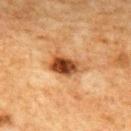Q: Was a biopsy performed?
A: no biopsy performed (imaged during a skin exam)
Q: Lesion size?
A: about 4 mm
Q: Patient demographics?
A: female, aged 58 to 62
Q: What kind of image is this?
A: ~15 mm crop, total-body skin-cancer survey
Q: Automated lesion metrics?
A: a footprint of about 8.5 mm² and two-axis asymmetry of about 0.3; an average lesion color of about L≈40 a*≈22 b*≈34 (CIELAB), a lesion–skin lightness drop of about 15, and a normalized border contrast of about 11.5; a border-irregularity index near 3/10, a color-variation rating of about 8/10, and radial color variation of about 2.5; a nevus-likeness score of about 80/100 and lesion-presence confidence of about 100/100
Q: Lesion location?
A: the upper back